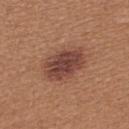Impression: The lesion was photographed on a routine skin check and not biopsied; there is no pathology result. Clinical summary: Imaged with white-light lighting. A female patient in their 40s. On the upper back. Cropped from a whole-body photographic skin survey; the tile spans about 15 mm.